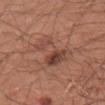Impression:
The lesion was tiled from a total-body skin photograph and was not biopsied.
Background:
A male subject about 30 years old. The lesion is on the right upper arm. The lesion's longest dimension is about 6.5 mm. Captured under white-light illumination. A region of skin cropped from a whole-body photographic capture, roughly 15 mm wide. Automated tile analysis of the lesion measured a border-irregularity index near 7/10, a color-variation rating of about 7.5/10, and peripheral color asymmetry of about 2.